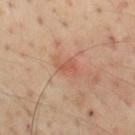<tbp_lesion>
  <automated_metrics>
    <cielab_L>56</cielab_L>
    <cielab_a>24</cielab_a>
    <cielab_b>32</cielab_b>
    <vs_skin_darker_L>7.0</vs_skin_darker_L>
    <vs_skin_contrast_norm>5.5</vs_skin_contrast_norm>
    <nevus_likeness_0_100>5</nevus_likeness_0_100>
    <lesion_detection_confidence_0_100>100</lesion_detection_confidence_0_100>
  </automated_metrics>
  <site>mid back</site>
  <image>
    <source>total-body photography crop</source>
    <field_of_view_mm>15</field_of_view_mm>
  </image>
  <lesion_size>
    <long_diameter_mm_approx>3.5</long_diameter_mm_approx>
  </lesion_size>
  <patient>
    <sex>male</sex>
    <age_approx>55</age_approx>
  </patient>
  <lighting>cross-polarized</lighting>
</tbp_lesion>